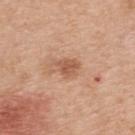Assessment:
Part of a total-body skin-imaging series; this lesion was reviewed on a skin check and was not flagged for biopsy.
Context:
Captured under white-light illumination. The subject is a male aged 68 to 72. Measured at roughly 3 mm in maximum diameter. A lesion tile, about 15 mm wide, cut from a 3D total-body photograph. The lesion is on the upper back. An algorithmic analysis of the crop reported a footprint of about 5 mm², a shape eccentricity near 0.65, and two-axis asymmetry of about 0.4. The software also gave a mean CIELAB color near L≈57 a*≈23 b*≈33, roughly 10 lightness units darker than nearby skin, and a normalized border contrast of about 7.